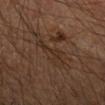• workup: catalogued during a skin exam; not biopsied
• site: the arm
• patient: male, aged approximately 40
• imaging modality: total-body-photography crop, ~15 mm field of view